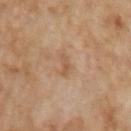  image:
    source: total-body photography crop
    field_of_view_mm: 15
  lighting: cross-polarized
  patient:
    sex: male
    age_approx: 65